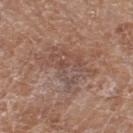Q: Was a biopsy performed?
A: imaged on a skin check; not biopsied
Q: Lesion location?
A: the right thigh
Q: What are the patient's age and sex?
A: female, in their mid- to late 70s
Q: What kind of image is this?
A: 15 mm crop, total-body photography
Q: What did automated image analysis measure?
A: a shape eccentricity near 0.85; a classifier nevus-likeness of about 0/100